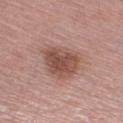Impression:
The lesion was photographed on a routine skin check and not biopsied; there is no pathology result.
Context:
The lesion is on the right lower leg. About 5.5 mm across. Captured under white-light illumination. A male patient, approximately 75 years of age. The lesion-visualizer software estimated an outline eccentricity of about 0.6 (0 = round, 1 = elongated) and a shape-asymmetry score of about 0.25 (0 = symmetric). The software also gave a lesion color around L≈51 a*≈21 b*≈25 in CIELAB, a lesion–skin lightness drop of about 11, and a normalized border contrast of about 8. The software also gave a border-irregularity index near 3/10 and internal color variation of about 4.5 on a 0–10 scale. The analysis additionally found an automated nevus-likeness rating near 35 out of 100 and a lesion-detection confidence of about 100/100. A close-up tile cropped from a whole-body skin photograph, about 15 mm across.